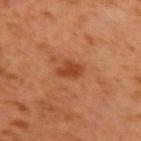| feature | finding |
|---|---|
| image source | ~15 mm crop, total-body skin-cancer survey |
| tile lighting | cross-polarized |
| patient | male, approximately 60 years of age |
| anatomic site | the left upper arm |
| size | about 3 mm |